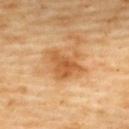{"biopsy_status": "not biopsied; imaged during a skin examination", "lesion_size": {"long_diameter_mm_approx": 4.5}, "lighting": "cross-polarized", "site": "upper back", "patient": {"sex": "female", "age_approx": 60}, "image": {"source": "total-body photography crop", "field_of_view_mm": 15}, "automated_metrics": {"area_mm2_approx": 12.0, "eccentricity": 0.65, "nevus_likeness_0_100": 10, "lesion_detection_confidence_0_100": 100}}